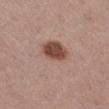{
  "patient": {
    "sex": "female",
    "age_approx": 55
  },
  "image": {
    "source": "total-body photography crop",
    "field_of_view_mm": 15
  },
  "lighting": "white-light",
  "automated_metrics": {
    "cielab_L": 54,
    "cielab_a": 18,
    "cielab_b": 24,
    "vs_skin_contrast_norm": 6.0,
    "border_irregularity_0_10": 3.0,
    "color_variation_0_10": 9.5,
    "peripheral_color_asymmetry": 3.0
  },
  "site": "left thigh"
}